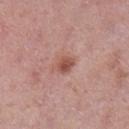Part of a total-body skin-imaging series; this lesion was reviewed on a skin check and was not flagged for biopsy.
This is a white-light tile.
The total-body-photography lesion software estimated a lesion area of about 4 mm², a shape eccentricity near 0.6, and a shape-asymmetry score of about 0.2 (0 = symmetric). And it measured a border-irregularity index near 2/10, a color-variation rating of about 3.5/10, and a peripheral color-asymmetry measure near 1. The analysis additionally found a lesion-detection confidence of about 100/100.
This image is a 15 mm lesion crop taken from a total-body photograph.
A female patient, approximately 50 years of age.
On the left lower leg.
Measured at roughly 2.5 mm in maximum diameter.